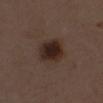Assessment:
Captured during whole-body skin photography for melanoma surveillance; the lesion was not biopsied.
Background:
The lesion is located on the chest. The lesion-visualizer software estimated an area of roughly 11 mm², a shape eccentricity near 0.4, and a symmetry-axis asymmetry near 0.15. A roughly 15 mm field-of-view crop from a total-body skin photograph. A female subject roughly 50 years of age. Longest diameter approximately 4 mm. This is a white-light tile.